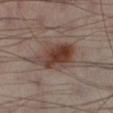Q: Was a biopsy performed?
A: total-body-photography surveillance lesion; no biopsy
Q: Automated lesion metrics?
A: border irregularity of about 2.5 on a 0–10 scale, a within-lesion color-variation index near 6/10, and radial color variation of about 2
Q: Lesion location?
A: the left lower leg
Q: What kind of image is this?
A: total-body-photography crop, ~15 mm field of view
Q: How was the tile lit?
A: cross-polarized
Q: Who is the patient?
A: male, aged around 40
Q: How large is the lesion?
A: about 6 mm